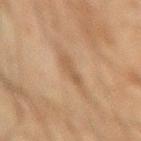{"image": {"source": "total-body photography crop", "field_of_view_mm": 15}, "patient": {"sex": "male", "age_approx": 45}, "site": "left forearm", "lighting": "cross-polarized"}